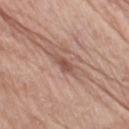Q: What kind of image is this?
A: total-body-photography crop, ~15 mm field of view
Q: How was the tile lit?
A: white-light illumination
Q: What are the patient's age and sex?
A: female, aged around 75
Q: Lesion location?
A: the left thigh